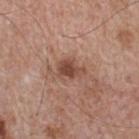Q: Patient demographics?
A: male, aged around 65
Q: What did automated image analysis measure?
A: a footprint of about 5 mm², an eccentricity of roughly 0.7, and a shape-asymmetry score of about 0.35 (0 = symmetric); a lesion color around L≈46 a*≈22 b*≈27 in CIELAB, roughly 11 lightness units darker than nearby skin, and a normalized border contrast of about 8.5; internal color variation of about 2.5 on a 0–10 scale and peripheral color asymmetry of about 1; an automated nevus-likeness rating near 75 out of 100 and lesion-presence confidence of about 100/100
Q: How large is the lesion?
A: about 3 mm
Q: What lighting was used for the tile?
A: white-light
Q: What kind of image is this?
A: ~15 mm tile from a whole-body skin photo
Q: Lesion location?
A: the upper back